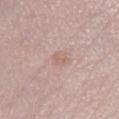No biopsy was performed on this lesion — it was imaged during a full skin examination and was not determined to be concerning. Approximately 1.5 mm at its widest. Automated tile analysis of the lesion measured an area of roughly 1.5 mm². The analysis additionally found a lesion color around L≈61 a*≈20 b*≈25 in CIELAB, a lesion–skin lightness drop of about 6, and a normalized lesion–skin contrast near 5. A close-up tile cropped from a whole-body skin photograph, about 15 mm across. Imaged with white-light lighting. A female subject, aged approximately 50. The lesion is located on the left lower leg.